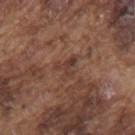{"image": {"source": "total-body photography crop", "field_of_view_mm": 15}, "patient": {"sex": "male", "age_approx": 75}, "site": "back", "lighting": "white-light"}